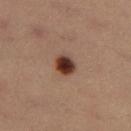The subject is a female aged 33 to 37. The lesion is on the leg. About 2.5 mm across. An algorithmic analysis of the crop reported an area of roughly 5 mm², an outline eccentricity of about 0.4 (0 = round, 1 = elongated), and two-axis asymmetry of about 0.15. This image is a 15 mm lesion crop taken from a total-body photograph.